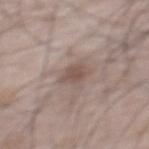Q: Is there a histopathology result?
A: total-body-photography surveillance lesion; no biopsy
Q: How was the tile lit?
A: white-light illumination
Q: How was this image acquired?
A: ~15 mm crop, total-body skin-cancer survey
Q: Lesion location?
A: the mid back
Q: What are the patient's age and sex?
A: male, roughly 65 years of age
Q: What is the lesion's diameter?
A: ≈3 mm